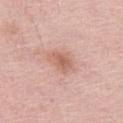image source: ~15 mm tile from a whole-body skin photo | tile lighting: white-light | lesion diameter: ~3.5 mm (longest diameter) | anatomic site: the left thigh | patient: female, in their mid- to late 60s.